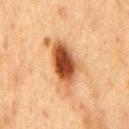biopsy_status: not biopsied; imaged during a skin examination
image:
  source: total-body photography crop
  field_of_view_mm: 15
site: chest
patient:
  sex: male
  age_approx: 75